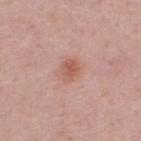No biopsy was performed on this lesion — it was imaged during a full skin examination and was not determined to be concerning. The patient is a male roughly 50 years of age. Cropped from a whole-body photographic skin survey; the tile spans about 15 mm. Located on the upper back.